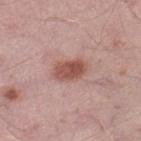Impression:
Captured during whole-body skin photography for melanoma surveillance; the lesion was not biopsied.
Background:
A male patient aged approximately 45. Located on the left thigh. A lesion tile, about 15 mm wide, cut from a 3D total-body photograph. Imaged with white-light lighting. Approximately 4 mm at its widest.